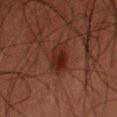Findings:
* follow-up: imaged on a skin check; not biopsied
* automated metrics: an area of roughly 8 mm², an eccentricity of roughly 0.55, and a shape-asymmetry score of about 0.2 (0 = symmetric); a border-irregularity index near 2/10, a color-variation rating of about 4.5/10, and a peripheral color-asymmetry measure near 1.5; an automated nevus-likeness rating near 95 out of 100 and a detector confidence of about 100 out of 100 that the crop contains a lesion
* patient: male, approximately 60 years of age
* lesion diameter: ~3.5 mm (longest diameter)
* imaging modality: ~15 mm tile from a whole-body skin photo
* body site: the arm